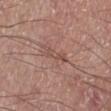<case>
<lesion_size>
  <long_diameter_mm_approx>4.0</long_diameter_mm_approx>
</lesion_size>
<patient>
  <sex>male</sex>
  <age_approx>55</age_approx>
</patient>
<site>left lower leg</site>
<image>
  <source>total-body photography crop</source>
  <field_of_view_mm>15</field_of_view_mm>
</image>
</case>